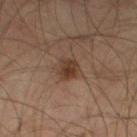| key | value |
|---|---|
| biopsy status | total-body-photography surveillance lesion; no biopsy |
| location | the left thigh |
| image | total-body-photography crop, ~15 mm field of view |
| diameter | about 3 mm |
| patient | male, in their mid- to late 50s |
| illumination | cross-polarized |